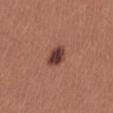Assessment:
Captured during whole-body skin photography for melanoma surveillance; the lesion was not biopsied.
Image and clinical context:
A 15 mm close-up tile from a total-body photography series done for melanoma screening. The lesion is on the right thigh. Measured at roughly 3 mm in maximum diameter. The patient is a female in their mid- to late 20s. The tile uses white-light illumination.